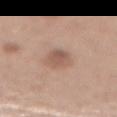Imaged during a routine full-body skin examination; the lesion was not biopsied and no histopathology is available. Automated image analysis of the tile measured a footprint of about 6.5 mm², an eccentricity of roughly 0.75, and two-axis asymmetry of about 0.15. The analysis additionally found an automated nevus-likeness rating near 45 out of 100 and a lesion-detection confidence of about 100/100. From the abdomen. Captured under white-light illumination. A roughly 15 mm field-of-view crop from a total-body skin photograph. About 3.5 mm across. The patient is a female about 40 years old.